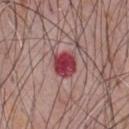Part of a total-body skin-imaging series; this lesion was reviewed on a skin check and was not flagged for biopsy. This is a white-light tile. The patient is a male aged around 60. About 3 mm across. The total-body-photography lesion software estimated an eccentricity of roughly 0.45 and a shape-asymmetry score of about 0.15 (0 = symmetric). And it measured roughly 16 lightness units darker than nearby skin. The analysis additionally found a border-irregularity index near 1.5/10 and a within-lesion color-variation index near 5/10. A region of skin cropped from a whole-body photographic capture, roughly 15 mm wide. From the chest.